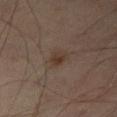biopsy_status: not biopsied; imaged during a skin examination